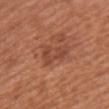<lesion>
  <biopsy_status>not biopsied; imaged during a skin examination</biopsy_status>
  <lesion_size>
    <long_diameter_mm_approx>4.0</long_diameter_mm_approx>
  </lesion_size>
  <image>
    <source>total-body photography crop</source>
    <field_of_view_mm>15</field_of_view_mm>
  </image>
  <lighting>white-light</lighting>
  <site>front of the torso</site>
  <patient>
    <sex>female</sex>
    <age_approx>60</age_approx>
  </patient>
</lesion>